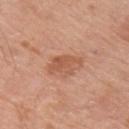Imaged during a routine full-body skin examination; the lesion was not biopsied and no histopathology is available.
The patient is a male aged 63 to 67.
A 15 mm close-up tile from a total-body photography series done for melanoma screening.
Captured under white-light illumination.
The lesion's longest dimension is about 3.5 mm.
The lesion-visualizer software estimated an area of roughly 6.5 mm², an outline eccentricity of about 0.75 (0 = round, 1 = elongated), and a shape-asymmetry score of about 0.35 (0 = symmetric). The analysis additionally found a mean CIELAB color near L≈56 a*≈25 b*≈33, roughly 9 lightness units darker than nearby skin, and a lesion-to-skin contrast of about 6 (normalized; higher = more distinct).
The lesion is located on the right upper arm.